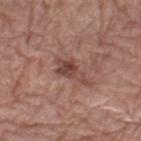Recorded during total-body skin imaging; not selected for excision or biopsy. The lesion's longest dimension is about 4.5 mm. Imaged with white-light lighting. On the left forearm. A male subject, aged approximately 70. Automated image analysis of the tile measured a lesion color around L≈45 a*≈21 b*≈24 in CIELAB, roughly 10 lightness units darker than nearby skin, and a normalized lesion–skin contrast near 7.5. The software also gave internal color variation of about 4.5 on a 0–10 scale and a peripheral color-asymmetry measure near 1.5. And it measured a nevus-likeness score of about 5/100 and a detector confidence of about 100 out of 100 that the crop contains a lesion. A lesion tile, about 15 mm wide, cut from a 3D total-body photograph.